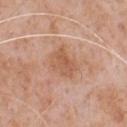body site = the chest; patient = male, aged around 65; lesion diameter = about 3.5 mm; image = 15 mm crop, total-body photography; illumination = white-light.